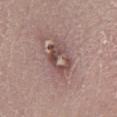Clinical summary:
On the left lower leg. The patient is a male in their mid-60s. A region of skin cropped from a whole-body photographic capture, roughly 15 mm wide.